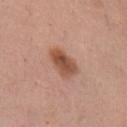biopsy status = total-body-photography surveillance lesion; no biopsy
diameter = about 4 mm
lighting = white-light
acquisition = total-body-photography crop, ~15 mm field of view
body site = the chest
patient = female, aged 28–32
image-analysis metrics = about 13 CIELAB-L* units darker than the surrounding skin and a normalized border contrast of about 9